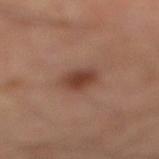Recorded during total-body skin imaging; not selected for excision or biopsy. This image is a 15 mm lesion crop taken from a total-body photograph. A male patient, roughly 50 years of age. Automated tile analysis of the lesion measured an area of roughly 6 mm², an eccentricity of roughly 0.65, and a symmetry-axis asymmetry near 0.2. The analysis additionally found a mean CIELAB color near L≈40 a*≈21 b*≈27, roughly 11 lightness units darker than nearby skin, and a normalized border contrast of about 9. It also reported a classifier nevus-likeness of about 90/100. The lesion is on the left leg. The recorded lesion diameter is about 3 mm. The tile uses cross-polarized illumination.